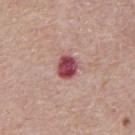Assessment:
Part of a total-body skin-imaging series; this lesion was reviewed on a skin check and was not flagged for biopsy.
Context:
This is a white-light tile. Cropped from a whole-body photographic skin survey; the tile spans about 15 mm. On the abdomen. The subject is a male about 65 years old. About 3 mm across.